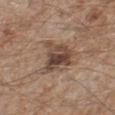Part of a total-body skin-imaging series; this lesion was reviewed on a skin check and was not flagged for biopsy. From the left lower leg. A male subject in their mid-60s. A 15 mm close-up extracted from a 3D total-body photography capture.